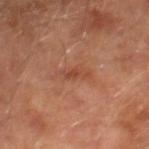follow-up = no biopsy performed (imaged during a skin exam) | anatomic site = the right lower leg | automated metrics = an eccentricity of roughly 0.95; a lesion color around L≈45 a*≈26 b*≈32 in CIELAB, a lesion–skin lightness drop of about 7, and a normalized lesion–skin contrast near 6; border irregularity of about 5.5 on a 0–10 scale | lesion diameter = ~3 mm (longest diameter) | subject = male, approximately 65 years of age | image source = ~15 mm tile from a whole-body skin photo.